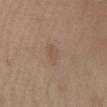workup: imaged on a skin check; not biopsied | location: the right lower leg | subject: male, in their 60s | TBP lesion metrics: an outline eccentricity of about 0.75 (0 = round, 1 = elongated) and a shape-asymmetry score of about 0.25 (0 = symmetric); a lesion color around L≈45 a*≈14 b*≈27 in CIELAB, about 5 CIELAB-L* units darker than the surrounding skin, and a normalized lesion–skin contrast near 5; a classifier nevus-likeness of about 0/100 and a lesion-detection confidence of about 100/100 | image source: ~15 mm crop, total-body skin-cancer survey | diameter: ~2.5 mm (longest diameter) | tile lighting: cross-polarized.